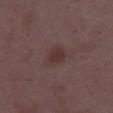Q: Was a biopsy performed?
A: no biopsy performed (imaged during a skin exam)
Q: What kind of image is this?
A: total-body-photography crop, ~15 mm field of view
Q: What is the lesion's diameter?
A: about 2.5 mm
Q: Patient demographics?
A: female, roughly 35 years of age
Q: Lesion location?
A: the right thigh